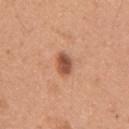Findings:
• biopsy status: imaged on a skin check; not biopsied
• site: the right upper arm
• size: ~2.5 mm (longest diameter)
• lighting: white-light illumination
• patient: female, aged around 45
• image source: 15 mm crop, total-body photography
• automated metrics: a footprint of about 4.5 mm² and an eccentricity of roughly 0.7; an average lesion color of about L≈52 a*≈25 b*≈32 (CIELAB) and roughly 14 lightness units darker than nearby skin; a border-irregularity rating of about 1.5/10, a color-variation rating of about 3.5/10, and peripheral color asymmetry of about 1